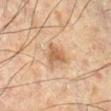Image and clinical context:
Approximately 2.5 mm at its widest. A male subject, in their 60s. A 15 mm close-up extracted from a 3D total-body photography capture. The lesion is located on the leg.